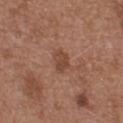Context: The lesion-visualizer software estimated a lesion area of about 4 mm² and a shape eccentricity near 0.65. The analysis additionally found a classifier nevus-likeness of about 20/100 and a detector confidence of about 100 out of 100 that the crop contains a lesion. A 15 mm close-up tile from a total-body photography series done for melanoma screening. Approximately 2.5 mm at its widest. Captured under white-light illumination. The subject is a female aged 38 to 42. From the upper back.